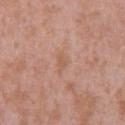Clinical impression:
Part of a total-body skin-imaging series; this lesion was reviewed on a skin check and was not flagged for biopsy.
Acquisition and patient details:
Imaged with white-light lighting. A female subject roughly 40 years of age. Located on the upper back. Longest diameter approximately 3 mm. A lesion tile, about 15 mm wide, cut from a 3D total-body photograph. An algorithmic analysis of the crop reported an area of roughly 3.5 mm², a shape eccentricity near 0.85, and a symmetry-axis asymmetry near 0.4. And it measured a mean CIELAB color near L≈58 a*≈21 b*≈30, about 5 CIELAB-L* units darker than the surrounding skin, and a normalized border contrast of about 4.5. And it measured a border-irregularity index near 3.5/10, a within-lesion color-variation index near 1/10, and peripheral color asymmetry of about 0.5. It also reported a classifier nevus-likeness of about 0/100 and lesion-presence confidence of about 100/100.